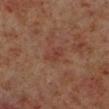Clinical impression:
The lesion was photographed on a routine skin check and not biopsied; there is no pathology result.
Acquisition and patient details:
This is a cross-polarized tile. Located on the left lower leg. A male patient, aged around 60. A lesion tile, about 15 mm wide, cut from a 3D total-body photograph. Approximately 3 mm at its widest. The lesion-visualizer software estimated an area of roughly 3.5 mm² and a shape eccentricity near 0.85. It also reported an automated nevus-likeness rating near 0 out of 100 and a detector confidence of about 100 out of 100 that the crop contains a lesion.